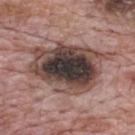Q: Was this lesion biopsied?
A: total-body-photography surveillance lesion; no biopsy
Q: Lesion location?
A: the upper back
Q: Illumination type?
A: white-light illumination
Q: What is the lesion's diameter?
A: ≈9.5 mm
Q: How was this image acquired?
A: ~15 mm tile from a whole-body skin photo
Q: Patient demographics?
A: male, in their 70s